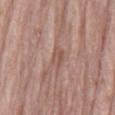Clinical impression:
Part of a total-body skin-imaging series; this lesion was reviewed on a skin check and was not flagged for biopsy.
Image and clinical context:
The tile uses white-light illumination. An algorithmic analysis of the crop reported an automated nevus-likeness rating near 0 out of 100 and lesion-presence confidence of about 60/100. The patient is a male in their 70s. A region of skin cropped from a whole-body photographic capture, roughly 15 mm wide. The recorded lesion diameter is about 2.5 mm. From the back.